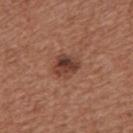Assessment:
Captured during whole-body skin photography for melanoma surveillance; the lesion was not biopsied.
Clinical summary:
A male patient, about 45 years old. Cropped from a total-body skin-imaging series; the visible field is about 15 mm. From the chest.